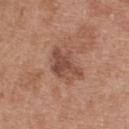biopsy_status: not biopsied; imaged during a skin examination
patient:
  sex: male
  age_approx: 30
image:
  source: total-body photography crop
  field_of_view_mm: 15
lesion_size:
  long_diameter_mm_approx: 5.0
lighting: white-light
site: upper back